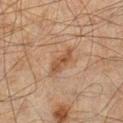The lesion was photographed on a routine skin check and not biopsied; there is no pathology result.
About 4 mm across.
The tile uses cross-polarized illumination.
A 15 mm crop from a total-body photograph taken for skin-cancer surveillance.
Located on the leg.
A male subject, roughly 45 years of age.
Automated tile analysis of the lesion measured a shape eccentricity near 0.9 and a shape-asymmetry score of about 0.35 (0 = symmetric). The analysis additionally found a mean CIELAB color near L≈41 a*≈17 b*≈28, a lesion–skin lightness drop of about 8, and a lesion-to-skin contrast of about 7 (normalized; higher = more distinct). The software also gave a border-irregularity index near 4/10 and internal color variation of about 2 on a 0–10 scale.